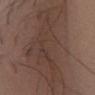Impression: Captured during whole-body skin photography for melanoma surveillance; the lesion was not biopsied. Acquisition and patient details: A close-up tile cropped from a whole-body skin photograph, about 15 mm across. The total-body-photography lesion software estimated an area of roughly 55 mm², a shape eccentricity near 0.9, and a shape-asymmetry score of about 0.5 (0 = symmetric). The software also gave a mean CIELAB color near L≈38 a*≈16 b*≈22, roughly 8 lightness units darker than nearby skin, and a normalized border contrast of about 7. The analysis additionally found a nevus-likeness score of about 0/100 and a detector confidence of about 55 out of 100 that the crop contains a lesion. On the right lower leg. This is a white-light tile. The patient is a male roughly 40 years of age. Approximately 13.5 mm at its widest.